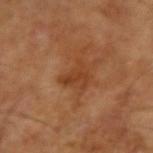  biopsy_status: not biopsied; imaged during a skin examination
  lesion_size:
    long_diameter_mm_approx: 3.0
  site: left arm
  image:
    source: total-body photography crop
    field_of_view_mm: 15
  lighting: cross-polarized
  patient:
    sex: male
    age_approx: 65